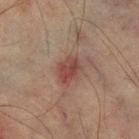<tbp_lesion>
<site>right lower leg</site>
<patient>
  <sex>male</sex>
  <age_approx>75</age_approx>
</patient>
<lesion_size>
  <long_diameter_mm_approx>3.0</long_diameter_mm_approx>
</lesion_size>
<lighting>cross-polarized</lighting>
<image>
  <source>total-body photography crop</source>
  <field_of_view_mm>15</field_of_view_mm>
</image>
</tbp_lesion>